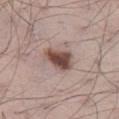Findings:
• follow-up: no biopsy performed (imaged during a skin exam)